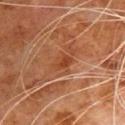<case>
<site>chest</site>
<image>
  <source>total-body photography crop</source>
  <field_of_view_mm>15</field_of_view_mm>
</image>
<patient>
  <sex>male</sex>
  <age_approx>80</age_approx>
</patient>
</case>